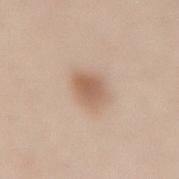Clinical impression:
The lesion was tiled from a total-body skin photograph and was not biopsied.
Acquisition and patient details:
A female patient in their 50s. From the lower back. A lesion tile, about 15 mm wide, cut from a 3D total-body photograph.